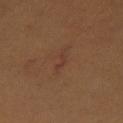The lesion was tiled from a total-body skin photograph and was not biopsied. A female patient about 45 years old. Automated tile analysis of the lesion measured a footprint of about 2 mm², an outline eccentricity of about 0.95 (0 = round, 1 = elongated), and two-axis asymmetry of about 0.55. It also reported a peripheral color-asymmetry measure near 0. The analysis additionally found a lesion-detection confidence of about 85/100. This is a cross-polarized tile. The recorded lesion diameter is about 3 mm. The lesion is on the right leg. A close-up tile cropped from a whole-body skin photograph, about 15 mm across.